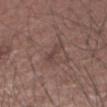Q: Was a biopsy performed?
A: imaged on a skin check; not biopsied
Q: Patient demographics?
A: male, aged 63 to 67
Q: How large is the lesion?
A: ~2.5 mm (longest diameter)
Q: Lesion location?
A: the right forearm
Q: Automated lesion metrics?
A: a footprint of about 2.5 mm², a shape eccentricity near 0.9, and a symmetry-axis asymmetry near 0.4; a lesion color around L≈41 a*≈18 b*≈20 in CIELAB, roughly 6 lightness units darker than nearby skin, and a normalized lesion–skin contrast near 5.5; a classifier nevus-likeness of about 0/100 and a detector confidence of about 90 out of 100 that the crop contains a lesion
Q: How was this image acquired?
A: total-body-photography crop, ~15 mm field of view